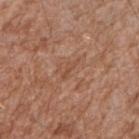Q: Was this lesion biopsied?
A: no biopsy performed (imaged during a skin exam)
Q: Illumination type?
A: white-light
Q: Lesion size?
A: ≈3 mm
Q: What did automated image analysis measure?
A: a footprint of about 2.5 mm², an outline eccentricity of about 0.95 (0 = round, 1 = elongated), and a shape-asymmetry score of about 0.5 (0 = symmetric); an average lesion color of about L≈49 a*≈22 b*≈31 (CIELAB), about 6 CIELAB-L* units darker than the surrounding skin, and a lesion-to-skin contrast of about 5 (normalized; higher = more distinct)
Q: Lesion location?
A: the right forearm
Q: What are the patient's age and sex?
A: male, aged 53–57
Q: How was this image acquired?
A: ~15 mm tile from a whole-body skin photo